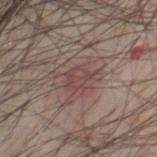biopsy status=total-body-photography surveillance lesion; no biopsy
subject=male, aged 58–62
lesion size=about 3 mm
imaging modality=15 mm crop, total-body photography
site=the front of the torso